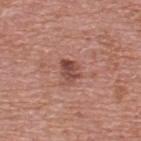Impression:
The lesion was photographed on a routine skin check and not biopsied; there is no pathology result.
Background:
The lesion is on the upper back. Approximately 3 mm at its widest. A male patient about 70 years old. A roughly 15 mm field-of-view crop from a total-body skin photograph. The tile uses white-light illumination.